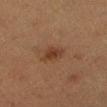Image and clinical context: The tile uses cross-polarized illumination. A female patient aged 38–42. The total-body-photography lesion software estimated an average lesion color of about L≈30 a*≈18 b*≈25 (CIELAB), about 7 CIELAB-L* units darker than the surrounding skin, and a lesion-to-skin contrast of about 7.5 (normalized; higher = more distinct). The software also gave a border-irregularity index near 3/10, a within-lesion color-variation index near 1.5/10, and a peripheral color-asymmetry measure near 0.5. A roughly 15 mm field-of-view crop from a total-body skin photograph. The lesion is on the left lower leg.